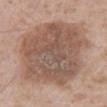lesion size = about 9.5 mm; tile lighting = white-light; subject = male, roughly 65 years of age; imaging modality = ~15 mm crop, total-body skin-cancer survey; anatomic site = the abdomen.